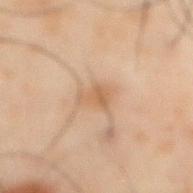Clinical impression: The lesion was tiled from a total-body skin photograph and was not biopsied. Background: An algorithmic analysis of the crop reported roughly 6 lightness units darker than nearby skin and a normalized border contrast of about 6. The analysis additionally found a border-irregularity index near 5/10, internal color variation of about 2 on a 0–10 scale, and a peripheral color-asymmetry measure near 0.5. The analysis additionally found a classifier nevus-likeness of about 5/100 and a lesion-detection confidence of about 100/100. A male subject about 50 years old. A close-up tile cropped from a whole-body skin photograph, about 15 mm across. Located on the abdomen. The recorded lesion diameter is about 3 mm.